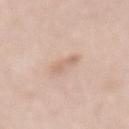No biopsy was performed on this lesion — it was imaged during a full skin examination and was not determined to be concerning.
Automated tile analysis of the lesion measured roughly 8 lightness units darker than nearby skin and a normalized lesion–skin contrast near 5. And it measured an automated nevus-likeness rating near 0 out of 100 and lesion-presence confidence of about 100/100.
About 3.5 mm across.
From the front of the torso.
A lesion tile, about 15 mm wide, cut from a 3D total-body photograph.
The subject is a female roughly 65 years of age.